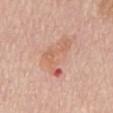Assessment: The lesion was photographed on a routine skin check and not biopsied; there is no pathology result. Context: The lesion's longest dimension is about 6 mm. A close-up tile cropped from a whole-body skin photograph, about 15 mm across. A female subject aged around 65. The total-body-photography lesion software estimated an eccentricity of roughly 0.9. The analysis additionally found a mean CIELAB color near L≈62 a*≈24 b*≈31, roughly 8 lightness units darker than nearby skin, and a normalized border contrast of about 6. On the back. This is a white-light tile.